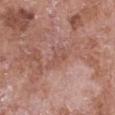<record>
<biopsy_status>not biopsied; imaged during a skin examination</biopsy_status>
<patient>
  <sex>female</sex>
  <age_approx>75</age_approx>
</patient>
<lighting>white-light</lighting>
<site>front of the torso</site>
<image>
  <source>total-body photography crop</source>
  <field_of_view_mm>15</field_of_view_mm>
</image>
<lesion_size>
  <long_diameter_mm_approx>3.5</long_diameter_mm_approx>
</lesion_size>
<automated_metrics>
  <area_mm2_approx>4.0</area_mm2_approx>
  <shape_asymmetry>0.4</shape_asymmetry>
  <cielab_L>52</cielab_L>
  <cielab_a>23</cielab_a>
  <cielab_b>26</cielab_b>
  <vs_skin_contrast_norm>5.0</vs_skin_contrast_norm>
</automated_metrics>
</record>